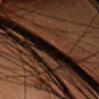Part of a total-body skin-imaging series; this lesion was reviewed on a skin check and was not flagged for biopsy.
The lesion is on the head or neck.
A female subject, aged 38–42.
A close-up tile cropped from a whole-body skin photograph, about 15 mm across.